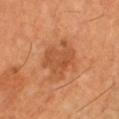Clinical impression: Part of a total-body skin-imaging series; this lesion was reviewed on a skin check and was not flagged for biopsy. Context: The tile uses cross-polarized illumination. A subject approximately 70 years of age. The lesion is on the right lower leg. Measured at roughly 5 mm in maximum diameter. This image is a 15 mm lesion crop taken from a total-body photograph.